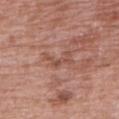workup=catalogued during a skin exam; not biopsied
lesion size=~3.5 mm (longest diameter)
image=15 mm crop, total-body photography
location=the right upper arm
tile lighting=white-light illumination
subject=female, roughly 70 years of age
automated lesion analysis=a lesion color around L≈51 a*≈23 b*≈27 in CIELAB and about 7 CIELAB-L* units darker than the surrounding skin